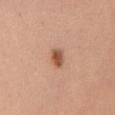Assessment: No biopsy was performed on this lesion — it was imaged during a full skin examination and was not determined to be concerning. Acquisition and patient details: A female patient, aged 48 to 52. The lesion is on the chest. A close-up tile cropped from a whole-body skin photograph, about 15 mm across.